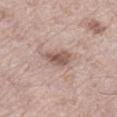follow-up = imaged on a skin check; not biopsied
subject = male, about 70 years old
anatomic site = the left thigh
acquisition = 15 mm crop, total-body photography